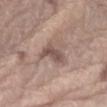{
  "biopsy_status": "not biopsied; imaged during a skin examination",
  "image": {
    "source": "total-body photography crop",
    "field_of_view_mm": 15
  },
  "site": "left forearm",
  "patient": {
    "sex": "male",
    "age_approx": 70
  }
}